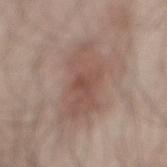Clinical impression:
No biopsy was performed on this lesion — it was imaged during a full skin examination and was not determined to be concerning.
Acquisition and patient details:
A lesion tile, about 15 mm wide, cut from a 3D total-body photograph. From the mid back. The lesion-visualizer software estimated a border-irregularity index near 4.5/10 and a color-variation rating of about 3.5/10. And it measured a classifier nevus-likeness of about 20/100. A male subject aged 43 to 47. About 8.5 mm across.